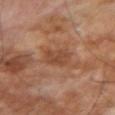Case summary:
• notes — catalogued during a skin exam; not biopsied
• image source — ~15 mm tile from a whole-body skin photo
• lesion diameter — ~3 mm (longest diameter)
• tile lighting — cross-polarized
• patient — male, aged 68–72
• automated lesion analysis — border irregularity of about 2.5 on a 0–10 scale, internal color variation of about 2 on a 0–10 scale, and peripheral color asymmetry of about 0.5; a classifier nevus-likeness of about 0/100 and a lesion-detection confidence of about 100/100
• body site — the right upper arm